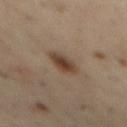- notes · catalogued during a skin exam; not biopsied
- lighting · cross-polarized illumination
- body site · the back
- image · total-body-photography crop, ~15 mm field of view
- lesion size · about 4 mm
- subject · male, aged approximately 55